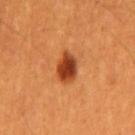biopsy_status: not biopsied; imaged during a skin examination
image:
  source: total-body photography crop
  field_of_view_mm: 15
patient:
  sex: male
  age_approx: 60
site: mid back
lesion_size:
  long_diameter_mm_approx: 4.0